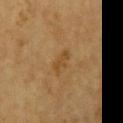Clinical impression:
Part of a total-body skin-imaging series; this lesion was reviewed on a skin check and was not flagged for biopsy.
Image and clinical context:
The patient is a male aged approximately 85 or older. A region of skin cropped from a whole-body photographic capture, roughly 15 mm wide. From the left upper arm. Captured under cross-polarized illumination.